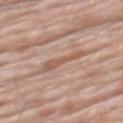No biopsy was performed on this lesion — it was imaged during a full skin examination and was not determined to be concerning. A male patient about 80 years old. This image is a 15 mm lesion crop taken from a total-body photograph. Longest diameter approximately 4 mm. Located on the mid back. This is a white-light tile.